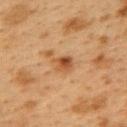Q: Was a biopsy performed?
A: imaged on a skin check; not biopsied
Q: Lesion size?
A: ≈2.5 mm
Q: What are the patient's age and sex?
A: female, roughly 40 years of age
Q: How was the tile lit?
A: cross-polarized
Q: Where on the body is the lesion?
A: the upper back
Q: How was this image acquired?
A: ~15 mm tile from a whole-body skin photo